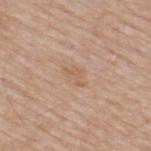This lesion was catalogued during total-body skin photography and was not selected for biopsy.
A male subject aged approximately 65.
On the upper back.
Cropped from a total-body skin-imaging series; the visible field is about 15 mm.